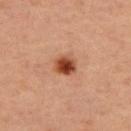Findings:
• biopsy status: imaged on a skin check; not biopsied
• image: 15 mm crop, total-body photography
• location: the upper back
• subject: female, in their mid-40s
• tile lighting: cross-polarized
• diameter: ≈2.5 mm